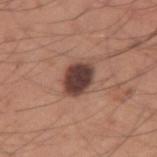| field | value |
|---|---|
| follow-up | imaged on a skin check; not biopsied |
| anatomic site | the right upper arm |
| patient | male, about 35 years old |
| acquisition | total-body-photography crop, ~15 mm field of view |
| illumination | white-light |